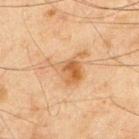Imaged during a routine full-body skin examination; the lesion was not biopsied and no histopathology is available. The recorded lesion diameter is about 5 mm. A roughly 15 mm field-of-view crop from a total-body skin photograph. The lesion is on the upper back. The subject is a male aged around 45. The lesion-visualizer software estimated a shape-asymmetry score of about 0.6 (0 = symmetric). And it measured a lesion color around L≈51 a*≈18 b*≈34 in CIELAB and a normalized lesion–skin contrast near 7.